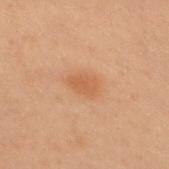Clinical impression: No biopsy was performed on this lesion — it was imaged during a full skin examination and was not determined to be concerning. Context: A lesion tile, about 15 mm wide, cut from a 3D total-body photograph. A male patient, aged 53 to 57. From the right upper arm.